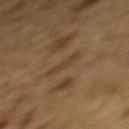notes: no biopsy performed (imaged during a skin exam) | automated lesion analysis: an area of roughly 3 mm², an outline eccentricity of about 0.95 (0 = round, 1 = elongated), and a symmetry-axis asymmetry near 0.3; an average lesion color of about L≈34 a*≈12 b*≈27 (CIELAB) and a normalized lesion–skin contrast near 5 | lesion size: ≈3 mm | tile lighting: cross-polarized illumination | subject: male, in their mid-80s or older | image source: ~15 mm crop, total-body skin-cancer survey | body site: the mid back.